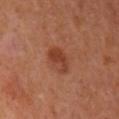| key | value |
|---|---|
| workup | catalogued during a skin exam; not biopsied |
| subject | male, in their mid-60s |
| acquisition | ~15 mm crop, total-body skin-cancer survey |
| site | the mid back |
| diameter | about 3.5 mm |
| illumination | cross-polarized illumination |
| automated metrics | border irregularity of about 2 on a 0–10 scale, a within-lesion color-variation index near 3.5/10, and radial color variation of about 1.5; a classifier nevus-likeness of about 90/100 and a detector confidence of about 100 out of 100 that the crop contains a lesion |